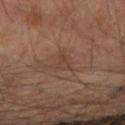Captured during whole-body skin photography for melanoma surveillance; the lesion was not biopsied. A patient aged 53–57. A roughly 15 mm field-of-view crop from a total-body skin photograph. Imaged with cross-polarized lighting. Located on the right forearm. Measured at roughly 3 mm in maximum diameter.